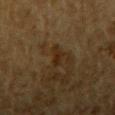<tbp_lesion>
<biopsy_status>not biopsied; imaged during a skin examination</biopsy_status>
<patient>
  <sex>male</sex>
  <age_approx>85+</age_approx>
</patient>
<lighting>cross-polarized</lighting>
<lesion_size>
  <long_diameter_mm_approx>2.5</long_diameter_mm_approx>
</lesion_size>
<image>
  <source>total-body photography crop</source>
  <field_of_view_mm>15</field_of_view_mm>
</image>
<automated_metrics>
  <vs_skin_contrast_norm>8.0</vs_skin_contrast_norm>
</automated_metrics>
<site>right upper arm</site>
</tbp_lesion>